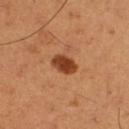Case summary:
• image source · 15 mm crop, total-body photography
• image-analysis metrics · a footprint of about 5.5 mm², an eccentricity of roughly 0.8, and a symmetry-axis asymmetry near 0.2; a mean CIELAB color near L≈42 a*≈27 b*≈37, a lesion–skin lightness drop of about 15, and a normalized border contrast of about 11.5; a border-irregularity index near 2/10, internal color variation of about 3 on a 0–10 scale, and peripheral color asymmetry of about 1
• tile lighting · cross-polarized
• diameter · ≈3.5 mm
• site · the right thigh
• subject · male, aged 48 to 52